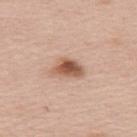<tbp_lesion>
  <biopsy_status>not biopsied; imaged during a skin examination</biopsy_status>
  <patient>
    <sex>female</sex>
    <age_approx>65</age_approx>
  </patient>
  <lesion_size>
    <long_diameter_mm_approx>3.5</long_diameter_mm_approx>
  </lesion_size>
  <lighting>white-light</lighting>
  <image>
    <source>total-body photography crop</source>
    <field_of_view_mm>15</field_of_view_mm>
  </image>
  <site>upper back</site>
</tbp_lesion>